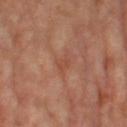biopsy status=imaged on a skin check; not biopsied
body site=the right leg
acquisition=15 mm crop, total-body photography
subject=female, aged 63–67
automated metrics=a lesion area of about 3 mm² and a shape eccentricity near 0.8; an average lesion color of about L≈46 a*≈24 b*≈30 (CIELAB) and a normalized border contrast of about 4.5; an automated nevus-likeness rating near 0 out of 100 and a detector confidence of about 100 out of 100 that the crop contains a lesion
lighting=cross-polarized illumination